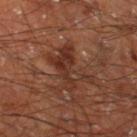workup: total-body-photography surveillance lesion; no biopsy
anatomic site: the left thigh
size: about 5.5 mm
patient: male, roughly 60 years of age
image-analysis metrics: a lesion area of about 11 mm², a shape eccentricity near 0.8, and a shape-asymmetry score of about 0.4 (0 = symmetric); a border-irregularity rating of about 7.5/10, a within-lesion color-variation index near 5/10, and peripheral color asymmetry of about 2; a nevus-likeness score of about 0/100
acquisition: total-body-photography crop, ~15 mm field of view